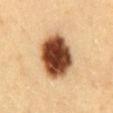No biopsy was performed on this lesion — it was imaged during a full skin examination and was not determined to be concerning. This is a cross-polarized tile. A female patient, aged around 30. Cropped from a total-body skin-imaging series; the visible field is about 15 mm. The lesion is located on the chest.